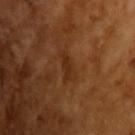Findings:
- subject: male, aged approximately 65
- acquisition: ~15 mm crop, total-body skin-cancer survey